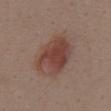Part of a total-body skin-imaging series; this lesion was reviewed on a skin check and was not flagged for biopsy. A female patient, approximately 55 years of age. A lesion tile, about 15 mm wide, cut from a 3D total-body photograph. On the front of the torso.